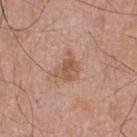The lesion was tiled from a total-body skin photograph and was not biopsied. A male patient aged 63–67. From the back. A roughly 15 mm field-of-view crop from a total-body skin photograph. The tile uses white-light illumination.